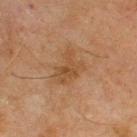Captured during whole-body skin photography for melanoma surveillance; the lesion was not biopsied.